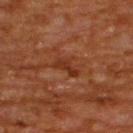follow-up — catalogued during a skin exam; not biopsied
size — ~3 mm (longest diameter)
patient — male, in their mid- to late 60s
acquisition — ~15 mm tile from a whole-body skin photo
lighting — cross-polarized
site — the back
TBP lesion metrics — a border-irregularity rating of about 4/10, a color-variation rating of about 1.5/10, and peripheral color asymmetry of about 0.5; a lesion-detection confidence of about 100/100